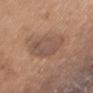• acquisition — total-body-photography crop, ~15 mm field of view
• anatomic site — the chest
• illumination — white-light illumination
• patient — female, approximately 65 years of age
• diameter — ~6.5 mm (longest diameter)